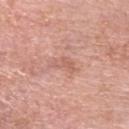Q: Was a biopsy performed?
A: catalogued during a skin exam; not biopsied
Q: What lighting was used for the tile?
A: white-light
Q: Lesion size?
A: about 3 mm
Q: Automated lesion metrics?
A: an outline eccentricity of about 0.85 (0 = round, 1 = elongated) and a shape-asymmetry score of about 0.45 (0 = symmetric); a lesion color around L≈61 a*≈24 b*≈28 in CIELAB, roughly 7 lightness units darker than nearby skin, and a lesion-to-skin contrast of about 5 (normalized; higher = more distinct); a within-lesion color-variation index near 2/10 and radial color variation of about 0.5; a nevus-likeness score of about 0/100 and a detector confidence of about 100 out of 100 that the crop contains a lesion
Q: Where on the body is the lesion?
A: the head or neck
Q: Who is the patient?
A: male, roughly 80 years of age
Q: How was this image acquired?
A: ~15 mm crop, total-body skin-cancer survey